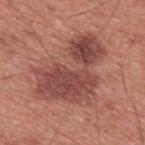{"biopsy_status": "not biopsied; imaged during a skin examination", "image": {"source": "total-body photography crop", "field_of_view_mm": 15}, "site": "upper back", "patient": {"sex": "male", "age_approx": 60}}